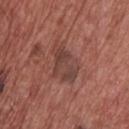Clinical impression:
The lesion was tiled from a total-body skin photograph and was not biopsied.
Context:
The lesion is on the upper back. A 15 mm close-up tile from a total-body photography series done for melanoma screening. The subject is a male approximately 70 years of age. Measured at roughly 5 mm in maximum diameter.